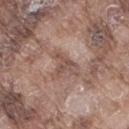Q: Is there a histopathology result?
A: imaged on a skin check; not biopsied
Q: Where on the body is the lesion?
A: the right thigh
Q: What is the imaging modality?
A: ~15 mm crop, total-body skin-cancer survey
Q: What did automated image analysis measure?
A: a shape eccentricity near 0.75 and a symmetry-axis asymmetry near 0.35; an average lesion color of about L≈50 a*≈18 b*≈24 (CIELAB), roughly 9 lightness units darker than nearby skin, and a normalized lesion–skin contrast near 6.5; a border-irregularity rating of about 5.5/10, a within-lesion color-variation index near 1.5/10, and peripheral color asymmetry of about 0.5
Q: Illumination type?
A: white-light illumination
Q: How large is the lesion?
A: ~3.5 mm (longest diameter)
Q: Who is the patient?
A: male, aged 73 to 77